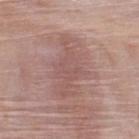Part of a total-body skin-imaging series; this lesion was reviewed on a skin check and was not flagged for biopsy.
The patient is a male approximately 50 years of age.
Measured at roughly 7 mm in maximum diameter.
The lesion is on the upper back.
The tile uses white-light illumination.
A close-up tile cropped from a whole-body skin photograph, about 15 mm across.
Automated image analysis of the tile measured an area of roughly 20 mm² and an outline eccentricity of about 0.85 (0 = round, 1 = elongated).